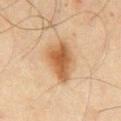workup = total-body-photography surveillance lesion; no biopsy
lesion size = ~5 mm (longest diameter)
patient = male, approximately 45 years of age
image source = total-body-photography crop, ~15 mm field of view
site = the chest